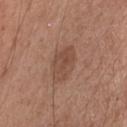Assessment:
The lesion was photographed on a routine skin check and not biopsied; there is no pathology result.
Acquisition and patient details:
A roughly 15 mm field-of-view crop from a total-body skin photograph. The subject is a male in their mid-50s. The total-body-photography lesion software estimated a lesion area of about 8 mm², an eccentricity of roughly 0.8, and a shape-asymmetry score of about 0.2 (0 = symmetric). The analysis additionally found an average lesion color of about L≈47 a*≈20 b*≈28 (CIELAB) and a lesion–skin lightness drop of about 8. Located on the chest. Measured at roughly 4.5 mm in maximum diameter.